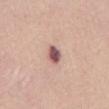Q: Was a biopsy performed?
A: imaged on a skin check; not biopsied
Q: What lighting was used for the tile?
A: white-light illumination
Q: What is the imaging modality?
A: ~15 mm tile from a whole-body skin photo
Q: What is the anatomic site?
A: the abdomen
Q: Automated lesion metrics?
A: an eccentricity of roughly 0.65 and a shape-asymmetry score of about 0.15 (0 = symmetric)
Q: How large is the lesion?
A: ≈2.5 mm
Q: Who is the patient?
A: female, aged approximately 35